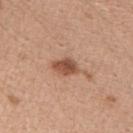  biopsy_status: not biopsied; imaged during a skin examination
  site: right upper arm
  patient:
    sex: female
    age_approx: 45
  image:
    source: total-body photography crop
    field_of_view_mm: 15
  lighting: white-light
  lesion_size:
    long_diameter_mm_approx: 3.0